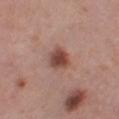Impression: Part of a total-body skin-imaging series; this lesion was reviewed on a skin check and was not flagged for biopsy. Context: A female subject aged 38–42. The lesion is on the left thigh. The lesion-visualizer software estimated a footprint of about 6.5 mm² and a shape-asymmetry score of about 0.25 (0 = symmetric). The analysis additionally found a lesion color around L≈46 a*≈22 b*≈25 in CIELAB, a lesion–skin lightness drop of about 13, and a lesion-to-skin contrast of about 9.5 (normalized; higher = more distinct). Cropped from a whole-body photographic skin survey; the tile spans about 15 mm. Imaged with white-light lighting.